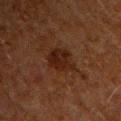workup — total-body-photography surveillance lesion; no biopsy
location — the front of the torso
subject — male, aged around 60
imaging modality — ~15 mm tile from a whole-body skin photo